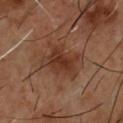biopsy_status: not biopsied; imaged during a skin examination
automated_metrics:
  area_mm2_approx: 14.0
  eccentricity: 0.6
  shape_asymmetry: 0.35
  cielab_L: 34
  cielab_a: 20
  cielab_b: 28
  vs_skin_contrast_norm: 7.5
patient:
  sex: male
  age_approx: 55
image:
  source: total-body photography crop
  field_of_view_mm: 15
site: chest